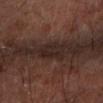Notes:
• notes · catalogued during a skin exam; not biopsied
• subject · male, roughly 65 years of age
• diameter · ~3 mm (longest diameter)
• anatomic site · the left forearm
• image · ~15 mm crop, total-body skin-cancer survey
• lighting · cross-polarized